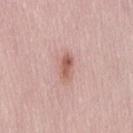biopsy status=imaged on a skin check; not biopsied
imaging modality=~15 mm tile from a whole-body skin photo
subject=female, about 40 years old
site=the lower back
image-analysis metrics=a border-irregularity rating of about 4/10, internal color variation of about 2.5 on a 0–10 scale, and peripheral color asymmetry of about 0.5